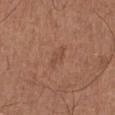Captured during whole-body skin photography for melanoma surveillance; the lesion was not biopsied. On the right lower leg. Measured at roughly 3 mm in maximum diameter. A male patient aged 73–77. Cropped from a whole-body photographic skin survey; the tile spans about 15 mm.